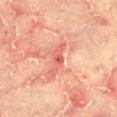Q: Was this lesion biopsied?
A: total-body-photography surveillance lesion; no biopsy
Q: Where on the body is the lesion?
A: the right thigh
Q: Patient demographics?
A: male, aged 63 to 67
Q: Lesion size?
A: about 4 mm
Q: What lighting was used for the tile?
A: cross-polarized
Q: How was this image acquired?
A: 15 mm crop, total-body photography